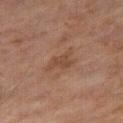Image and clinical context: On the right thigh. A male patient in their 60s. Automated image analysis of the tile measured an area of roughly 5 mm², a shape eccentricity near 0.8, and a shape-asymmetry score of about 0.45 (0 = symmetric). The analysis additionally found an automated nevus-likeness rating near 0 out of 100 and a detector confidence of about 100 out of 100 that the crop contains a lesion. Cropped from a total-body skin-imaging series; the visible field is about 15 mm.